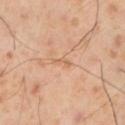Notes:
* workup — catalogued during a skin exam; not biopsied
* anatomic site — the left lower leg
* lesion size — about 2.5 mm
* lighting — cross-polarized
* image — ~15 mm tile from a whole-body skin photo
* patient — male, aged around 55